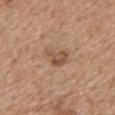Part of a total-body skin-imaging series; this lesion was reviewed on a skin check and was not flagged for biopsy. The lesion is located on the mid back. The tile uses white-light illumination. A region of skin cropped from a whole-body photographic capture, roughly 15 mm wide. Automated image analysis of the tile measured about 10 CIELAB-L* units darker than the surrounding skin and a normalized lesion–skin contrast near 7. And it measured a lesion-detection confidence of about 100/100. A male patient, roughly 70 years of age.